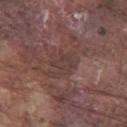Captured during whole-body skin photography for melanoma surveillance; the lesion was not biopsied. Approximately 2.5 mm at its widest. From the left thigh. A male patient in their mid-70s. Cropped from a total-body skin-imaging series; the visible field is about 15 mm. Automated tile analysis of the lesion measured a lesion area of about 3.5 mm², a shape eccentricity near 0.7, and a symmetry-axis asymmetry near 0.45. The analysis additionally found a lesion color around L≈38 a*≈17 b*≈19 in CIELAB and a lesion-to-skin contrast of about 5 (normalized; higher = more distinct). The analysis additionally found border irregularity of about 4.5 on a 0–10 scale, internal color variation of about 1.5 on a 0–10 scale, and peripheral color asymmetry of about 0.5. It also reported an automated nevus-likeness rating near 0 out of 100.